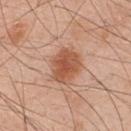follow-up=imaged on a skin check; not biopsied | imaging modality=15 mm crop, total-body photography | anatomic site=the chest | patient=male, about 50 years old.